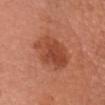| key | value |
|---|---|
| workup | imaged on a skin check; not biopsied |
| lesion diameter | ≈6 mm |
| automated lesion analysis | an area of roughly 16 mm² |
| image source | total-body-photography crop, ~15 mm field of view |
| anatomic site | the chest |
| subject | female, in their mid-60s |